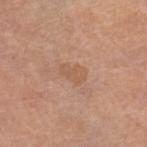Q: Was this lesion biopsied?
A: total-body-photography surveillance lesion; no biopsy
Q: What are the patient's age and sex?
A: female, approximately 65 years of age
Q: Lesion location?
A: the left leg
Q: How was this image acquired?
A: 15 mm crop, total-body photography